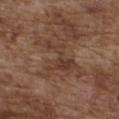The lesion was photographed on a routine skin check and not biopsied; there is no pathology result.
The lesion's longest dimension is about 5 mm.
A male subject approximately 75 years of age.
A lesion tile, about 15 mm wide, cut from a 3D total-body photograph.
Imaged with white-light lighting.
The lesion is on the chest.